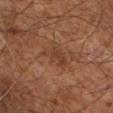* notes: no biopsy performed (imaged during a skin exam)
* illumination: cross-polarized illumination
* acquisition: 15 mm crop, total-body photography
* location: the right lower leg
* subject: aged 63 to 67